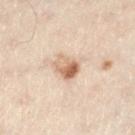Image and clinical context:
A 15 mm close-up tile from a total-body photography series done for melanoma screening. From the left lower leg. A male patient, roughly 50 years of age. Approximately 3.5 mm at its widest. This is a cross-polarized tile.